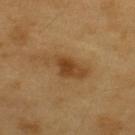A male patient, about 60 years old.
This is a cross-polarized tile.
Automated tile analysis of the lesion measured an area of roughly 10 mm² and an eccentricity of roughly 0.9. The software also gave a lesion color around L≈43 a*≈18 b*≈36 in CIELAB and a normalized lesion–skin contrast near 7.5. The analysis additionally found a border-irregularity rating of about 3.5/10 and a color-variation rating of about 3.5/10. The software also gave a classifier nevus-likeness of about 35/100.
From the back.
This image is a 15 mm lesion crop taken from a total-body photograph.
The lesion's longest dimension is about 5.5 mm.